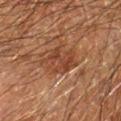Findings:
* follow-up — no biopsy performed (imaged during a skin exam)
* image source — ~15 mm tile from a whole-body skin photo
* subject — male, in their 60s
* location — the left forearm